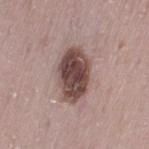Clinical impression: The lesion was photographed on a routine skin check and not biopsied; there is no pathology result. Acquisition and patient details: A region of skin cropped from a whole-body photographic capture, roughly 15 mm wide. The patient is a female in their 30s. Located on the left thigh.